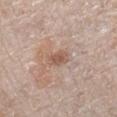Assessment:
No biopsy was performed on this lesion — it was imaged during a full skin examination and was not determined to be concerning.
Acquisition and patient details:
Located on the left forearm. A region of skin cropped from a whole-body photographic capture, roughly 15 mm wide. A female patient approximately 65 years of age. This is a white-light tile.